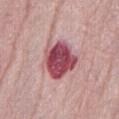{"biopsy_status": "not biopsied; imaged during a skin examination"}